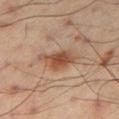Q: What is the anatomic site?
A: the right thigh
Q: How was the tile lit?
A: cross-polarized illumination
Q: What are the patient's age and sex?
A: male, in their mid-50s
Q: How was this image acquired?
A: ~15 mm tile from a whole-body skin photo
Q: Automated lesion metrics?
A: a lesion area of about 9.5 mm², an eccentricity of roughly 0.85, and a shape-asymmetry score of about 0.4 (0 = symmetric); roughly 11 lightness units darker than nearby skin and a normalized lesion–skin contrast near 8.5